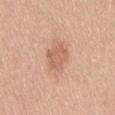* biopsy status · total-body-photography surveillance lesion; no biopsy
* location · the back
* automated metrics · a lesion area of about 8 mm², a shape eccentricity near 0.75, and two-axis asymmetry of about 0.25; a border-irregularity index near 2.5/10, a within-lesion color-variation index near 2.5/10, and a peripheral color-asymmetry measure near 1; a nevus-likeness score of about 55/100
* lesion size · ≈4 mm
* subject · female, approximately 55 years of age
* image source · total-body-photography crop, ~15 mm field of view
* lighting · white-light illumination